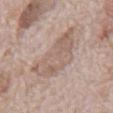workup: catalogued during a skin exam; not biopsied